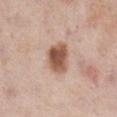  biopsy_status: not biopsied; imaged during a skin examination
  patient:
    sex: female
    age_approx: 65
  lesion_size:
    long_diameter_mm_approx: 4.0
  image:
    source: total-body photography crop
    field_of_view_mm: 15
  lighting: white-light
  site: right lower leg